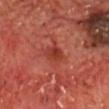Q: Is there a histopathology result?
A: total-body-photography surveillance lesion; no biopsy
Q: What is the lesion's diameter?
A: ≈4 mm
Q: Lesion location?
A: the head or neck
Q: How was this image acquired?
A: 15 mm crop, total-body photography
Q: How was the tile lit?
A: cross-polarized illumination
Q: Patient demographics?
A: male, roughly 70 years of age